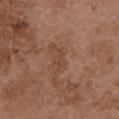Q: Was a biopsy performed?
A: total-body-photography surveillance lesion; no biopsy
Q: Lesion location?
A: the front of the torso
Q: What did automated image analysis measure?
A: a footprint of about 3 mm², an eccentricity of roughly 0.85, and two-axis asymmetry of about 0.45
Q: How was this image acquired?
A: ~15 mm tile from a whole-body skin photo
Q: Patient demographics?
A: female, in their mid- to late 60s
Q: Lesion size?
A: ≈2.5 mm
Q: How was the tile lit?
A: white-light illumination